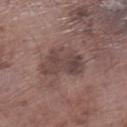Imaged during a routine full-body skin examination; the lesion was not biopsied and no histopathology is available.
On the left lower leg.
A male subject, aged around 75.
This is a white-light tile.
A 15 mm close-up extracted from a 3D total-body photography capture.
Measured at roughly 5 mm in maximum diameter.
The total-body-photography lesion software estimated a footprint of about 12 mm², a shape eccentricity near 0.8, and two-axis asymmetry of about 0.3. And it measured internal color variation of about 4 on a 0–10 scale and radial color variation of about 1.